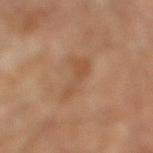workup = total-body-photography surveillance lesion; no biopsy
lighting = cross-polarized
TBP lesion metrics = an eccentricity of roughly 0.75 and a symmetry-axis asymmetry near 0.45; a mean CIELAB color near L≈50 a*≈19 b*≈33, about 6 CIELAB-L* units darker than the surrounding skin, and a normalized lesion–skin contrast near 5; border irregularity of about 5 on a 0–10 scale and radial color variation of about 1; a detector confidence of about 100 out of 100 that the crop contains a lesion
subject = aged approximately 55
image = ~15 mm tile from a whole-body skin photo
body site = the right lower leg